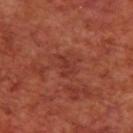biopsy status — catalogued during a skin exam; not biopsied
location — the back
image-analysis metrics — a mean CIELAB color near L≈34 a*≈28 b*≈28 and a lesion-to-skin contrast of about 5 (normalized; higher = more distinct); a classifier nevus-likeness of about 0/100 and lesion-presence confidence of about 100/100
image source — total-body-photography crop, ~15 mm field of view
lesion size — ~2.5 mm (longest diameter)
tile lighting — cross-polarized
subject — male, aged approximately 70